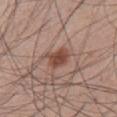Part of a total-body skin-imaging series; this lesion was reviewed on a skin check and was not flagged for biopsy.
The recorded lesion diameter is about 3 mm.
The lesion is on the abdomen.
The tile uses white-light illumination.
An algorithmic analysis of the crop reported a footprint of about 6 mm², a shape eccentricity near 0.65, and a shape-asymmetry score of about 0.3 (0 = symmetric).
A 15 mm close-up extracted from a 3D total-body photography capture.
A male subject, roughly 45 years of age.